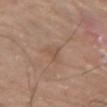notes: total-body-photography surveillance lesion; no biopsy
patient: male, aged around 80
body site: the arm
diameter: ≈3.5 mm
imaging modality: 15 mm crop, total-body photography